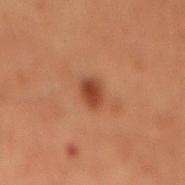Assessment:
Recorded during total-body skin imaging; not selected for excision or biopsy.
Background:
A roughly 15 mm field-of-view crop from a total-body skin photograph. A male patient roughly 60 years of age. The lesion is located on the mid back. Captured under cross-polarized illumination. About 2.5 mm across.